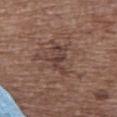The lesion was photographed on a routine skin check and not biopsied; there is no pathology result.
The lesion-visualizer software estimated a border-irregularity rating of about 7.5/10, a color-variation rating of about 3.5/10, and radial color variation of about 1. The analysis additionally found an automated nevus-likeness rating near 10 out of 100 and a lesion-detection confidence of about 90/100.
This is a white-light tile.
The lesion's longest dimension is about 4.5 mm.
The lesion is on the upper back.
A female patient roughly 75 years of age.
Cropped from a whole-body photographic skin survey; the tile spans about 15 mm.